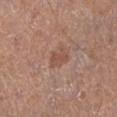workup — catalogued during a skin exam; not biopsied | tile lighting — white-light | lesion size — ~2.5 mm (longest diameter) | location — the left lower leg | acquisition — ~15 mm tile from a whole-body skin photo | patient — male, approximately 60 years of age.